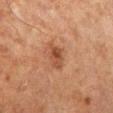biopsy status=catalogued during a skin exam; not biopsied
subject=male, in their mid- to late 60s
illumination=cross-polarized
TBP lesion metrics=a mean CIELAB color near L≈38 a*≈21 b*≈28, roughly 9 lightness units darker than nearby skin, and a normalized lesion–skin contrast near 7.5; a border-irregularity index near 2.5/10, internal color variation of about 4 on a 0–10 scale, and peripheral color asymmetry of about 1.5; a nevus-likeness score of about 40/100 and lesion-presence confidence of about 100/100
body site=the left lower leg
image source=15 mm crop, total-body photography